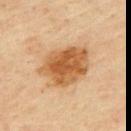| field | value |
|---|---|
| workup | catalogued during a skin exam; not biopsied |
| subject | male, roughly 45 years of age |
| site | the back |
| image-analysis metrics | an average lesion color of about L≈49 a*≈20 b*≈36 (CIELAB) and about 12 CIELAB-L* units darker than the surrounding skin; internal color variation of about 4.5 on a 0–10 scale and peripheral color asymmetry of about 1.5; a nevus-likeness score of about 95/100 and lesion-presence confidence of about 100/100 |
| image source | ~15 mm tile from a whole-body skin photo |
| lesion size | about 6.5 mm |